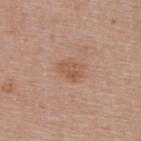Image and clinical context: The subject is a female aged 48–52. The lesion's longest dimension is about 2.5 mm. Cropped from a total-body skin-imaging series; the visible field is about 15 mm. The lesion is located on the upper back.